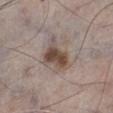Clinical impression:
Captured during whole-body skin photography for melanoma surveillance; the lesion was not biopsied.